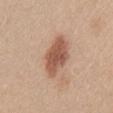The lesion was photographed on a routine skin check and not biopsied; there is no pathology result.
The tile uses white-light illumination.
The lesion's longest dimension is about 5.5 mm.
This image is a 15 mm lesion crop taken from a total-body photograph.
From the abdomen.
The subject is a male aged 28 to 32.
Automated image analysis of the tile measured a lesion area of about 13 mm² and an outline eccentricity of about 0.85 (0 = round, 1 = elongated). The software also gave a lesion-to-skin contrast of about 9 (normalized; higher = more distinct). The analysis additionally found border irregularity of about 2.5 on a 0–10 scale, a within-lesion color-variation index near 3/10, and a peripheral color-asymmetry measure near 1. The analysis additionally found a lesion-detection confidence of about 100/100.